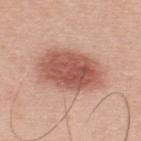Recorded during total-body skin imaging; not selected for excision or biopsy. A close-up tile cropped from a whole-body skin photograph, about 15 mm across. Longest diameter approximately 7.5 mm. The lesion is on the upper back. An algorithmic analysis of the crop reported an average lesion color of about L≈55 a*≈25 b*≈28 (CIELAB) and roughly 14 lightness units darker than nearby skin. A male patient about 45 years old.